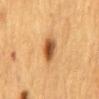Impression: Part of a total-body skin-imaging series; this lesion was reviewed on a skin check and was not flagged for biopsy. Clinical summary: Approximately 3.5 mm at its widest. The tile uses cross-polarized illumination. The subject is a female in their mid-50s. From the mid back. A close-up tile cropped from a whole-body skin photograph, about 15 mm across.